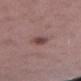Findings:
– workup · no biopsy performed (imaged during a skin exam)
– acquisition · ~15 mm crop, total-body skin-cancer survey
– site · the left thigh
– size · about 3 mm
– subject · male, in their mid-50s
– tile lighting · white-light